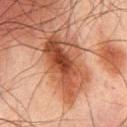Assessment:
Imaged during a routine full-body skin examination; the lesion was not biopsied and no histopathology is available.
Acquisition and patient details:
On the mid back. The tile uses cross-polarized illumination. Approximately 8 mm at its widest. A male patient aged approximately 65. Cropped from a whole-body photographic skin survey; the tile spans about 15 mm.